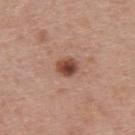Part of a total-body skin-imaging series; this lesion was reviewed on a skin check and was not flagged for biopsy. A 15 mm close-up tile from a total-body photography series done for melanoma screening. A male patient aged around 55. The tile uses white-light illumination. About 2.5 mm across.